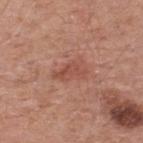workup: imaged on a skin check; not biopsied
TBP lesion metrics: lesion-presence confidence of about 100/100
acquisition: ~15 mm tile from a whole-body skin photo
subject: male, approximately 55 years of age
lesion diameter: about 4 mm
anatomic site: the back